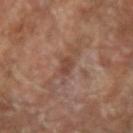Part of a total-body skin-imaging series; this lesion was reviewed on a skin check and was not flagged for biopsy. Cropped from a whole-body photographic skin survey; the tile spans about 15 mm. A male subject, in their mid- to late 60s. On the arm.